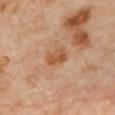This lesion was catalogued during total-body skin photography and was not selected for biopsy. A 15 mm close-up extracted from a 3D total-body photography capture. This is a cross-polarized tile. From the back. A female subject, aged around 60.